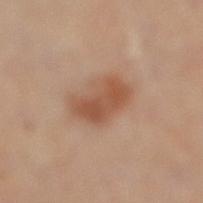follow-up = catalogued during a skin exam; not biopsied
automated lesion analysis = an average lesion color of about L≈53 a*≈22 b*≈32 (CIELAB), a lesion–skin lightness drop of about 10, and a normalized lesion–skin contrast near 7.5; a border-irregularity index near 3/10 and internal color variation of about 4 on a 0–10 scale
acquisition = ~15 mm crop, total-body skin-cancer survey
subject = male, aged around 65
tile lighting = cross-polarized illumination
location = the leg
diameter = about 5 mm